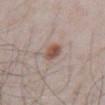Clinical impression:
Imaged during a routine full-body skin examination; the lesion was not biopsied and no histopathology is available.
Background:
A male patient, approximately 60 years of age. Automated tile analysis of the lesion measured a lesion color around L≈52 a*≈19 b*≈25 in CIELAB, roughly 12 lightness units darker than nearby skin, and a normalized border contrast of about 9. The analysis additionally found a border-irregularity index near 1.5/10, a color-variation rating of about 4.5/10, and peripheral color asymmetry of about 1.5. It also reported a lesion-detection confidence of about 100/100. Located on the abdomen. A region of skin cropped from a whole-body photographic capture, roughly 15 mm wide.